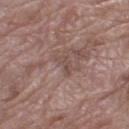workup: catalogued during a skin exam; not biopsied
body site: the left thigh
subject: female, aged 68 to 72
image source: ~15 mm tile from a whole-body skin photo